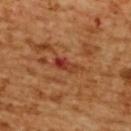Part of a total-body skin-imaging series; this lesion was reviewed on a skin check and was not flagged for biopsy. This is a cross-polarized tile. A female subject, aged 53 to 57. Located on the upper back. A roughly 15 mm field-of-view crop from a total-body skin photograph.